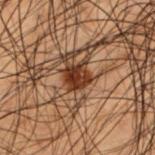| key | value |
|---|---|
| workup | catalogued during a skin exam; not biopsied |
| image | total-body-photography crop, ~15 mm field of view |
| patient | male, aged approximately 50 |
| lesion diameter | ~3 mm (longest diameter) |
| body site | the upper back |
| illumination | cross-polarized |